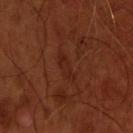<record>
<biopsy_status>not biopsied; imaged during a skin examination</biopsy_status>
<image>
  <source>total-body photography crop</source>
  <field_of_view_mm>15</field_of_view_mm>
</image>
<site>head or neck</site>
<automated_metrics>
  <border_irregularity_0_10>4.0</border_irregularity_0_10>
  <color_variation_0_10>1.5</color_variation_0_10>
  <peripheral_color_asymmetry>0.5</peripheral_color_asymmetry>
</automated_metrics>
<patient>
  <sex>male</sex>
  <age_approx>55</age_approx>
</patient>
<lesion_size>
  <long_diameter_mm_approx>4.5</long_diameter_mm_approx>
</lesion_size>
<lighting>cross-polarized</lighting>
</record>